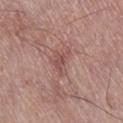Clinical impression:
Imaged during a routine full-body skin examination; the lesion was not biopsied and no histopathology is available.
Image and clinical context:
This image is a 15 mm lesion crop taken from a total-body photograph. On the right lower leg. The patient is a male aged around 55. This is a white-light tile. Longest diameter approximately 3.5 mm.